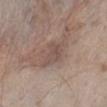<tbp_lesion>
  <biopsy_status>not biopsied; imaged during a skin examination</biopsy_status>
  <lesion_size>
    <long_diameter_mm_approx>3.0</long_diameter_mm_approx>
  </lesion_size>
  <automated_metrics>
    <vs_skin_darker_L>7.0</vs_skin_darker_L>
    <vs_skin_contrast_norm>5.0</vs_skin_contrast_norm>
  </automated_metrics>
  <image>
    <source>total-body photography crop</source>
    <field_of_view_mm>15</field_of_view_mm>
  </image>
  <lighting>white-light</lighting>
  <patient>
    <sex>male</sex>
    <age_approx>60</age_approx>
  </patient>
  <site>left lower leg</site>
</tbp_lesion>